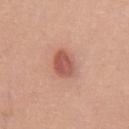| feature | finding |
|---|---|
| workup | catalogued during a skin exam; not biopsied |
| tile lighting | white-light illumination |
| image source | ~15 mm crop, total-body skin-cancer survey |
| location | the front of the torso |
| patient | female, aged approximately 40 |
| size | about 3.5 mm |